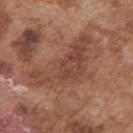workup=imaged on a skin check; not biopsied
anatomic site=the arm
patient=male, aged 73 to 77
tile lighting=white-light illumination
lesion diameter=≈9 mm
imaging modality=~15 mm crop, total-body skin-cancer survey